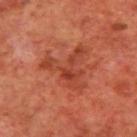workup: imaged on a skin check; not biopsied
location: the upper back
lighting: cross-polarized illumination
imaging modality: 15 mm crop, total-body photography
subject: male, aged approximately 70